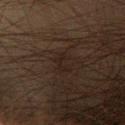The lesion was tiled from a total-body skin photograph and was not biopsied. An algorithmic analysis of the crop reported a footprint of about 2.5 mm², an eccentricity of roughly 0.85, and a symmetry-axis asymmetry near 0.4. The software also gave border irregularity of about 6 on a 0–10 scale and a within-lesion color-variation index near 0/10. Measured at roughly 2.5 mm in maximum diameter. Located on the chest. A male subject, aged around 65. A roughly 15 mm field-of-view crop from a total-body skin photograph.